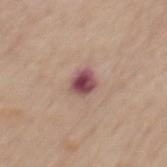A close-up tile cropped from a whole-body skin photograph, about 15 mm across. The lesion is located on the mid back. The total-body-photography lesion software estimated roughly 15 lightness units darker than nearby skin and a normalized border contrast of about 11.5. The software also gave a border-irregularity index near 2/10. The subject is a male in their mid-60s.